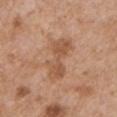Impression:
This lesion was catalogued during total-body skin photography and was not selected for biopsy.
Clinical summary:
The lesion is located on the left upper arm. An algorithmic analysis of the crop reported a shape eccentricity near 0.9 and a symmetry-axis asymmetry near 0.3. The software also gave an automated nevus-likeness rating near 0 out of 100 and a detector confidence of about 100 out of 100 that the crop contains a lesion. The subject is a male about 65 years old. A 15 mm crop from a total-body photograph taken for skin-cancer surveillance. Approximately 5.5 mm at its widest. The tile uses white-light illumination.